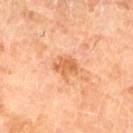The lesion was photographed on a routine skin check and not biopsied; there is no pathology result. On the left lower leg. A 15 mm close-up extracted from a 3D total-body photography capture. This is a cross-polarized tile. A female patient in their 70s.